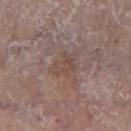<tbp_lesion>
<biopsy_status>not biopsied; imaged during a skin examination</biopsy_status>
<image>
  <source>total-body photography crop</source>
  <field_of_view_mm>15</field_of_view_mm>
</image>
<patient>
  <sex>female</sex>
  <age_approx>85+</age_approx>
</patient>
<site>right lower leg</site>
<lighting>white-light</lighting>
</tbp_lesion>